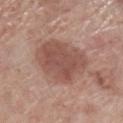{
  "biopsy_status": "not biopsied; imaged during a skin examination",
  "patient": {
    "sex": "male",
    "age_approx": 70
  },
  "image": {
    "source": "total-body photography crop",
    "field_of_view_mm": 15
  },
  "site": "left lower leg",
  "lighting": "white-light",
  "automated_metrics": {
    "area_mm2_approx": 25.0,
    "shape_asymmetry": 0.2,
    "vs_skin_contrast_norm": 7.5,
    "nevus_likeness_0_100": 10,
    "lesion_detection_confidence_0_100": 100
  },
  "lesion_size": {
    "long_diameter_mm_approx": 6.0
  }
}